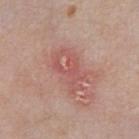<lesion>
  <biopsy_status>not biopsied; imaged during a skin examination</biopsy_status>
  <lesion_size>
    <long_diameter_mm_approx>5.0</long_diameter_mm_approx>
  </lesion_size>
  <site>chest</site>
  <patient>
    <sex>male</sex>
    <age_approx>80</age_approx>
  </patient>
  <image>
    <source>total-body photography crop</source>
    <field_of_view_mm>15</field_of_view_mm>
  </image>
</lesion>